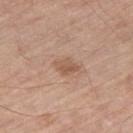  biopsy_status: not biopsied; imaged during a skin examination
  lesion_size:
    long_diameter_mm_approx: 3.0
  image:
    source: total-body photography crop
    field_of_view_mm: 15
  automated_metrics:
    cielab_L: 55
    cielab_a: 20
    cielab_b: 30
    vs_skin_darker_L: 9.0
    vs_skin_contrast_norm: 6.0
    color_variation_0_10: 2.5
    peripheral_color_asymmetry: 1.0
  site: right thigh
  lighting: white-light
  patient:
    sex: male
    age_approx: 70